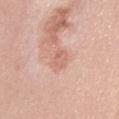The lesion was photographed on a routine skin check and not biopsied; there is no pathology result.
The tile uses white-light illumination.
Approximately 3 mm at its widest.
An algorithmic analysis of the crop reported border irregularity of about 3 on a 0–10 scale and a within-lesion color-variation index near 2.5/10.
A close-up tile cropped from a whole-body skin photograph, about 15 mm across.
From the back.
A female patient roughly 40 years of age.